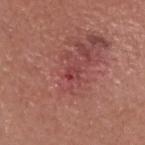  site: head or neck
  image:
    source: total-body photography crop
    field_of_view_mm: 15
  patient:
    sex: male
    age_approx: 45
  lighting: white-light
  automated_metrics:
    area_mm2_approx: 2.0
    eccentricity: 0.7
    shape_asymmetry: 0.3
    lesion_detection_confidence_0_100: 100
  diagnosis:
    histopathology: nodular basal cell carcinoma
    malignancy: malignant
    taxonomic_path:
      - Malignant
      - Malignant adnexal epithelial proliferations - Follicular
      - Basal cell carcinoma
      - Basal cell carcinoma, Nodular